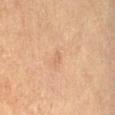Findings:
* workup · imaged on a skin check; not biopsied
* location · the right thigh
* subject · female, approximately 65 years of age
* lighting · cross-polarized illumination
* image source · 15 mm crop, total-body photography